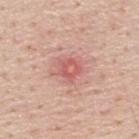{
  "biopsy_status": "not biopsied; imaged during a skin examination",
  "site": "upper back",
  "patient": {
    "sex": "male",
    "age_approx": 60
  },
  "image": {
    "source": "total-body photography crop",
    "field_of_view_mm": 15
  },
  "lesion_size": {
    "long_diameter_mm_approx": 2.5
  },
  "automated_metrics": {
    "area_mm2_approx": 4.5,
    "shape_asymmetry": 0.25,
    "color_variation_0_10": 3.5,
    "peripheral_color_asymmetry": 1.0,
    "nevus_likeness_0_100": 0,
    "lesion_detection_confidence_0_100": 100
  }
}